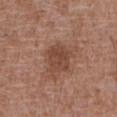From the abdomen. Automated tile analysis of the lesion measured a nevus-likeness score of about 10/100 and a detector confidence of about 100 out of 100 that the crop contains a lesion. Approximately 4 mm at its widest. Imaged with white-light lighting. A male patient, aged approximately 75. Cropped from a whole-body photographic skin survey; the tile spans about 15 mm.